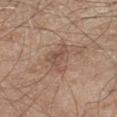This lesion was catalogued during total-body skin photography and was not selected for biopsy. A male subject aged approximately 60. An algorithmic analysis of the crop reported a footprint of about 6.5 mm². The software also gave a mean CIELAB color near L≈51 a*≈18 b*≈27 and a lesion-to-skin contrast of about 6 (normalized; higher = more distinct). The software also gave a color-variation rating of about 3.5/10 and peripheral color asymmetry of about 1. And it measured an automated nevus-likeness rating near 0 out of 100 and lesion-presence confidence of about 100/100. On the left lower leg. The tile uses white-light illumination. Approximately 4 mm at its widest. A 15 mm close-up tile from a total-body photography series done for melanoma screening.